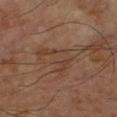Impression: Part of a total-body skin-imaging series; this lesion was reviewed on a skin check and was not flagged for biopsy. Background: From the arm. A region of skin cropped from a whole-body photographic capture, roughly 15 mm wide. A male patient, approximately 70 years of age.